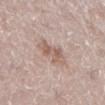Notes:
• notes — imaged on a skin check; not biopsied
• tile lighting — white-light illumination
• body site — the leg
• patient — female, aged 48–52
• imaging modality — ~15 mm tile from a whole-body skin photo